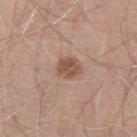| feature | finding |
|---|---|
| location | the left upper arm |
| image source | ~15 mm tile from a whole-body skin photo |
| patient | male, aged 68–72 |